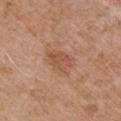follow-up: catalogued during a skin exam; not biopsied | patient: male, in their mid-70s | image source: 15 mm crop, total-body photography | lesion diameter: ~3.5 mm (longest diameter) | illumination: white-light illumination | location: the right upper arm.